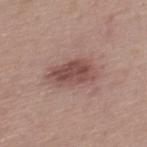• size — ≈5 mm
• location — the upper back
• subject — female, aged 48 to 52
• acquisition — 15 mm crop, total-body photography
• illumination — white-light